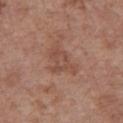follow-up = imaged on a skin check; not biopsied
lighting = white-light
subject = female, about 75 years old
image-analysis metrics = a lesion area of about 7.5 mm² and an eccentricity of roughly 0.75; a mean CIELAB color near L≈48 a*≈21 b*≈27, a lesion–skin lightness drop of about 7, and a normalized border contrast of about 5; border irregularity of about 6 on a 0–10 scale and a peripheral color-asymmetry measure near 1
body site = the chest
size = about 4.5 mm
imaging modality = 15 mm crop, total-body photography